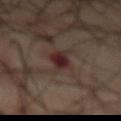patient:
  sex: male
  age_approx: 70
lesion_size:
  long_diameter_mm_approx: 4.5
image:
  source: total-body photography crop
  field_of_view_mm: 15
automated_metrics:
  eccentricity: 0.9
  shape_asymmetry: 0.35
  cielab_L: 26
  cielab_a: 17
  cielab_b: 16
  vs_skin_darker_L: 9.0
  vs_skin_contrast_norm: 10.0
  lesion_detection_confidence_0_100: 100
site: front of the torso
lighting: cross-polarized